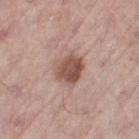biopsy status = imaged on a skin check; not biopsied | lesion size = ≈4 mm | acquisition = 15 mm crop, total-body photography | site = the left thigh | illumination = white-light illumination | automated lesion analysis = a mean CIELAB color near L≈52 a*≈20 b*≈25, about 13 CIELAB-L* units darker than the surrounding skin, and a normalized border contrast of about 9; a border-irregularity rating of about 2/10, a color-variation rating of about 4.5/10, and radial color variation of about 1.5 | patient = male, aged approximately 55.